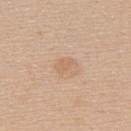follow-up = total-body-photography surveillance lesion; no biopsy | subject = female, approximately 45 years of age | site = the upper back | image = ~15 mm tile from a whole-body skin photo.